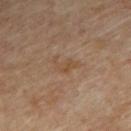Recorded during total-body skin imaging; not selected for excision or biopsy. The lesion's longest dimension is about 3 mm. Located on the upper back. A male subject, in their mid- to late 80s or older. Captured under cross-polarized illumination. A close-up tile cropped from a whole-body skin photograph, about 15 mm across.